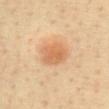Case summary:
- biopsy status — catalogued during a skin exam; not biopsied
- location — the abdomen
- patient — roughly 60 years of age
- illumination — cross-polarized illumination
- automated lesion analysis — a footprint of about 8.5 mm² and a shape-asymmetry score of about 0.15 (0 = symmetric)
- diameter — about 3.5 mm
- imaging modality — 15 mm crop, total-body photography